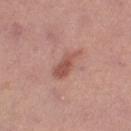notes — no biopsy performed (imaged during a skin exam) | location — the right thigh | diameter — ~4.5 mm (longest diameter) | tile lighting — white-light illumination | subject — female, aged approximately 40 | image — ~15 mm crop, total-body skin-cancer survey.